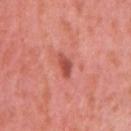<lesion>
  <biopsy_status>not biopsied; imaged during a skin examination</biopsy_status>
  <patient>
    <sex>female</sex>
    <age_approx>40</age_approx>
  </patient>
  <image>
    <source>total-body photography crop</source>
    <field_of_view_mm>15</field_of_view_mm>
  </image>
  <automated_metrics>
    <area_mm2_approx>3.5</area_mm2_approx>
    <eccentricity>0.85</eccentricity>
    <shape_asymmetry>0.3</shape_asymmetry>
    <color_variation_0_10>1.5</color_variation_0_10>
  </automated_metrics>
  <lesion_size>
    <long_diameter_mm_approx>3.0</long_diameter_mm_approx>
  </lesion_size>
  <site>right upper arm</site>
</lesion>